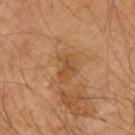workup: total-body-photography surveillance lesion; no biopsy